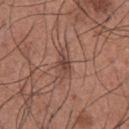Notes:
- biopsy status: catalogued during a skin exam; not biopsied
- acquisition: 15 mm crop, total-body photography
- patient: male, aged 33–37
- illumination: white-light
- site: the chest
- automated metrics: an average lesion color of about L≈45 a*≈20 b*≈25 (CIELAB) and a normalized lesion–skin contrast near 6.5; a border-irregularity rating of about 3.5/10 and radial color variation of about 1; a detector confidence of about 95 out of 100 that the crop contains a lesion